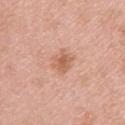| feature | finding |
|---|---|
| workup | catalogued during a skin exam; not biopsied |
| body site | the right upper arm |
| subject | male, aged approximately 60 |
| imaging modality | ~15 mm crop, total-body skin-cancer survey |